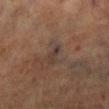| key | value |
|---|---|
| notes | imaged on a skin check; not biopsied |
| subject | male, approximately 70 years of age |
| location | the left lower leg |
| imaging modality | ~15 mm tile from a whole-body skin photo |